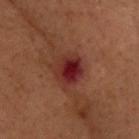The lesion was photographed on a routine skin check and not biopsied; there is no pathology result. On the head or neck. A male patient in their mid- to late 50s. A 15 mm close-up tile from a total-body photography series done for melanoma screening. The recorded lesion diameter is about 3.5 mm. Captured under cross-polarized illumination.